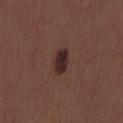follow-up = total-body-photography surveillance lesion; no biopsy | subject = male, in their 30s | lesion diameter = about 3 mm | location = the back | acquisition = 15 mm crop, total-body photography.